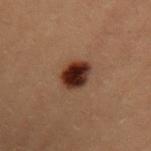Impression: Part of a total-body skin-imaging series; this lesion was reviewed on a skin check and was not flagged for biopsy. Image and clinical context: The subject is a female approximately 20 years of age. On the upper back. Cropped from a total-body skin-imaging series; the visible field is about 15 mm.